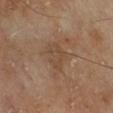Imaged during a routine full-body skin examination; the lesion was not biopsied and no histopathology is available. A lesion tile, about 15 mm wide, cut from a 3D total-body photograph. The lesion is on the right lower leg. Automated tile analysis of the lesion measured a lesion color around L≈46 a*≈16 b*≈29 in CIELAB, roughly 6 lightness units darker than nearby skin, and a lesion-to-skin contrast of about 5 (normalized; higher = more distinct). The patient is a male about 65 years old. The recorded lesion diameter is about 3 mm. This is a cross-polarized tile.